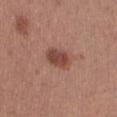– follow-up — no biopsy performed (imaged during a skin exam)